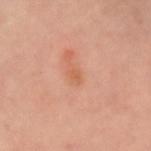Clinical impression: The lesion was tiled from a total-body skin photograph and was not biopsied. Clinical summary: On the left forearm. Longest diameter approximately 1 mm. A region of skin cropped from a whole-body photographic capture, roughly 15 mm wide. A female patient, aged 58–62. This is a cross-polarized tile.